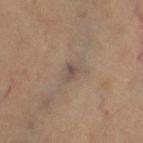Assessment: Captured during whole-body skin photography for melanoma surveillance; the lesion was not biopsied. Acquisition and patient details: From the right thigh. The total-body-photography lesion software estimated a mean CIELAB color near L≈41 a*≈11 b*≈19 and about 6 CIELAB-L* units darker than the surrounding skin. Longest diameter approximately 2.5 mm. Captured under cross-polarized illumination. A female subject roughly 70 years of age. This image is a 15 mm lesion crop taken from a total-body photograph.